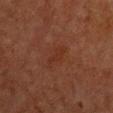A roughly 15 mm field-of-view crop from a total-body skin photograph. A female subject aged 53 to 57. Captured under cross-polarized illumination. The lesion's longest dimension is about 3.5 mm. Located on the front of the torso.